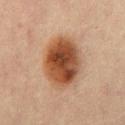Impression: Recorded during total-body skin imaging; not selected for excision or biopsy. Context: The subject is a male roughly 70 years of age. Cropped from a whole-body photographic skin survey; the tile spans about 15 mm. From the mid back.